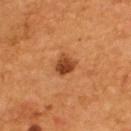Q: Is there a histopathology result?
A: no biopsy performed (imaged during a skin exam)
Q: How large is the lesion?
A: ~2.5 mm (longest diameter)
Q: What kind of image is this?
A: total-body-photography crop, ~15 mm field of view
Q: Where on the body is the lesion?
A: the upper back
Q: Patient demographics?
A: male, aged approximately 55
Q: What lighting was used for the tile?
A: cross-polarized
Q: Automated lesion metrics?
A: a footprint of about 5 mm² and an eccentricity of roughly 0.4; a border-irregularity index near 2.5/10, internal color variation of about 4 on a 0–10 scale, and a peripheral color-asymmetry measure near 1.5; a classifier nevus-likeness of about 95/100 and lesion-presence confidence of about 100/100